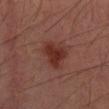patient=male, approximately 45 years of age; illumination=cross-polarized; image source=~15 mm tile from a whole-body skin photo; size=~4.5 mm (longest diameter); location=the mid back.